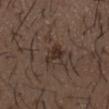The lesion was tiled from a total-body skin photograph and was not biopsied. A region of skin cropped from a whole-body photographic capture, roughly 15 mm wide. The subject is a male approximately 50 years of age. From the mid back.